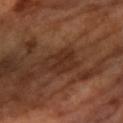biopsy_status: not biopsied; imaged during a skin examination
lighting: cross-polarized
site: arm
automated_metrics:
  eccentricity: 0.6
  vs_skin_darker_L: 6.0
  vs_skin_contrast_norm: 6.5
  border_irregularity_0_10: 3.0
  color_variation_0_10: 3.0
  peripheral_color_asymmetry: 1.0
  nevus_likeness_0_100: 0
  lesion_detection_confidence_0_100: 100
image:
  source: total-body photography crop
  field_of_view_mm: 15
patient:
  sex: female
  age_approx: 70
lesion_size:
  long_diameter_mm_approx: 4.0